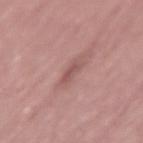workup=catalogued during a skin exam; not biopsied | diameter=~2.5 mm (longest diameter) | subject=male, in their 70s | body site=the left thigh | image source=~15 mm tile from a whole-body skin photo.